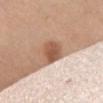Part of a total-body skin-imaging series; this lesion was reviewed on a skin check and was not flagged for biopsy. The patient is a female about 55 years old. Longest diameter approximately 3 mm. Located on the chest. Cropped from a whole-body photographic skin survey; the tile spans about 15 mm. Automated tile analysis of the lesion measured a footprint of about 8 mm², an eccentricity of roughly 0.4, and two-axis asymmetry of about 0.1. It also reported border irregularity of about 1 on a 0–10 scale, internal color variation of about 3.5 on a 0–10 scale, and peripheral color asymmetry of about 1. The analysis additionally found a classifier nevus-likeness of about 0/100.